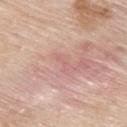Imaged during a routine full-body skin examination; the lesion was not biopsied and no histopathology is available. The subject is a male aged approximately 60. A roughly 15 mm field-of-view crop from a total-body skin photograph. From the upper back. About 2.5 mm across.